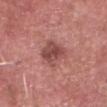Q: Was a biopsy performed?
A: catalogued during a skin exam; not biopsied
Q: What did automated image analysis measure?
A: a mean CIELAB color near L≈48 a*≈26 b*≈24, a lesion–skin lightness drop of about 11, and a normalized lesion–skin contrast near 7.5; internal color variation of about 4 on a 0–10 scale; an automated nevus-likeness rating near 10 out of 100 and a detector confidence of about 100 out of 100 that the crop contains a lesion
Q: What lighting was used for the tile?
A: white-light illumination
Q: Who is the patient?
A: male, aged approximately 80
Q: Where on the body is the lesion?
A: the head or neck
Q: What is the imaging modality?
A: 15 mm crop, total-body photography
Q: How large is the lesion?
A: ~3.5 mm (longest diameter)